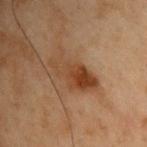biopsy status: catalogued during a skin exam; not biopsied
subject: male, in their mid-50s
site: the upper back
image: total-body-photography crop, ~15 mm field of view